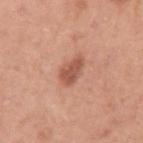{"biopsy_status": "not biopsied; imaged during a skin examination", "automated_metrics": {"area_mm2_approx": 5.5, "eccentricity": 0.7, "shape_asymmetry": 0.25, "cielab_L": 54, "cielab_a": 26, "cielab_b": 30, "vs_skin_darker_L": 12.0, "vs_skin_contrast_norm": 7.5, "nevus_likeness_0_100": 65, "lesion_detection_confidence_0_100": 100}, "patient": {"sex": "male", "age_approx": 50}, "image": {"source": "total-body photography crop", "field_of_view_mm": 15}, "lighting": "white-light", "site": "left upper arm", "lesion_size": {"long_diameter_mm_approx": 3.0}}